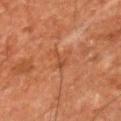biopsy_status: not biopsied; imaged during a skin examination
image:
  source: total-body photography crop
  field_of_view_mm: 15
site: arm
patient:
  sex: male
  age_approx: 75